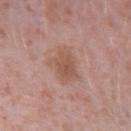The lesion was photographed on a routine skin check and not biopsied; there is no pathology result. The patient is a male about 40 years old. The lesion is located on the arm. Imaged with white-light lighting. Longest diameter approximately 3.5 mm. A 15 mm crop from a total-body photograph taken for skin-cancer surveillance.